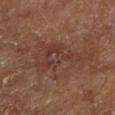{"biopsy_status": "not biopsied; imaged during a skin examination", "image": {"source": "total-body photography crop", "field_of_view_mm": 15}, "site": "left lower leg", "lesion_size": {"long_diameter_mm_approx": 8.0}, "patient": {"age_approx": 65}, "lighting": "cross-polarized"}